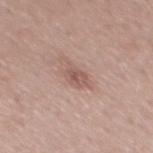| field | value |
|---|---|
| imaging modality | ~15 mm crop, total-body skin-cancer survey |
| location | the mid back |
| lesion size | ≈2.5 mm |
| subject | male, about 55 years old |
| illumination | white-light illumination |